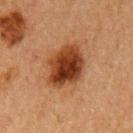follow-up = no biopsy performed (imaged during a skin exam); subject = female, about 60 years old; image source = ~15 mm crop, total-body skin-cancer survey; body site = the left upper arm; size = about 5 mm.